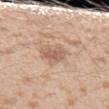  biopsy_status: not biopsied; imaged during a skin examination